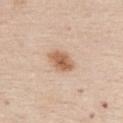Impression:
Imaged during a routine full-body skin examination; the lesion was not biopsied and no histopathology is available.
Clinical summary:
This is a white-light tile. The recorded lesion diameter is about 3.5 mm. The patient is a female aged 68–72. The lesion is on the chest. A 15 mm crop from a total-body photograph taken for skin-cancer surveillance.